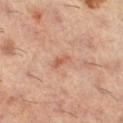Recorded during total-body skin imaging; not selected for excision or biopsy. A lesion tile, about 15 mm wide, cut from a 3D total-body photograph. A female subject, aged around 60. The tile uses cross-polarized illumination. The lesion-visualizer software estimated a border-irregularity index near 4.5/10, a color-variation rating of about 0/10, and a peripheral color-asymmetry measure near 0. From the right lower leg.